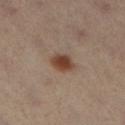Imaged during a routine full-body skin examination; the lesion was not biopsied and no histopathology is available.
A roughly 15 mm field-of-view crop from a total-body skin photograph.
Located on the left leg.
Measured at roughly 3 mm in maximum diameter.
The tile uses cross-polarized illumination.
The lesion-visualizer software estimated a symmetry-axis asymmetry near 0.2. The software also gave a lesion-detection confidence of about 100/100.
The patient is a female roughly 40 years of age.